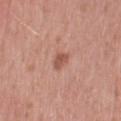Part of a total-body skin-imaging series; this lesion was reviewed on a skin check and was not flagged for biopsy.
The patient is a female about 70 years old.
A 15 mm crop from a total-body photograph taken for skin-cancer surveillance.
The lesion is located on the right thigh.